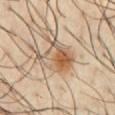This lesion was catalogued during total-body skin photography and was not selected for biopsy. From the chest. A male patient roughly 40 years of age. A close-up tile cropped from a whole-body skin photograph, about 15 mm across.